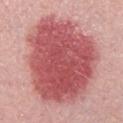Impression:
No biopsy was performed on this lesion — it was imaged during a full skin examination and was not determined to be concerning.
Acquisition and patient details:
Longest diameter approximately 12.5 mm. The lesion-visualizer software estimated a lesion area of about 75 mm² and two-axis asymmetry of about 0.15. The software also gave a color-variation rating of about 5/10 and radial color variation of about 1.5. This image is a 15 mm lesion crop taken from a total-body photograph. This is a white-light tile. The subject is a male roughly 30 years of age.